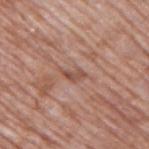{
  "biopsy_status": "not biopsied; imaged during a skin examination",
  "lighting": "white-light",
  "lesion_size": {
    "long_diameter_mm_approx": 3.0
  },
  "patient": {
    "sex": "male",
    "age_approx": 70
  },
  "site": "upper back",
  "image": {
    "source": "total-body photography crop",
    "field_of_view_mm": 15
  }
}